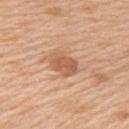Recorded during total-body skin imaging; not selected for excision or biopsy. The patient is a female approximately 45 years of age. Measured at roughly 3 mm in maximum diameter. The lesion-visualizer software estimated border irregularity of about 2 on a 0–10 scale, a within-lesion color-variation index near 2.5/10, and peripheral color asymmetry of about 1. And it measured a classifier nevus-likeness of about 80/100 and a lesion-detection confidence of about 100/100. The tile uses white-light illumination. On the left upper arm. This image is a 15 mm lesion crop taken from a total-body photograph.